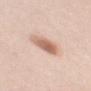biopsy_status: not biopsied; imaged during a skin examination
lesion_size:
  long_diameter_mm_approx: 4.0
patient:
  sex: female
  age_approx: 30
image:
  source: total-body photography crop
  field_of_view_mm: 15
site: mid back